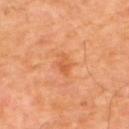This lesion was catalogued during total-body skin photography and was not selected for biopsy.
A 15 mm close-up tile from a total-body photography series done for melanoma screening.
A male subject aged around 55.
The recorded lesion diameter is about 3 mm.
The lesion is on the upper back.
Imaged with cross-polarized lighting.